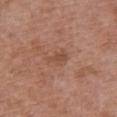Q: How was this image acquired?
A: ~15 mm tile from a whole-body skin photo
Q: Lesion size?
A: ≈2.5 mm
Q: What lighting was used for the tile?
A: white-light
Q: Who is the patient?
A: female, aged 73 to 77
Q: What is the anatomic site?
A: the chest